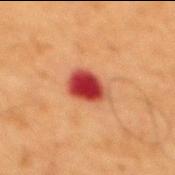The tile uses cross-polarized illumination. Located on the back. The lesion's longest dimension is about 4 mm. A region of skin cropped from a whole-body photographic capture, roughly 15 mm wide. An algorithmic analysis of the crop reported a border-irregularity rating of about 2/10 and a within-lesion color-variation index near 5.5/10. And it measured a nevus-likeness score of about 0/100 and lesion-presence confidence of about 100/100. The subject is a male aged around 65.